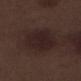Case summary:
– follow-up — total-body-photography surveillance lesion; no biopsy
– image — ~15 mm crop, total-body skin-cancer survey
– lesion size — about 5 mm
– subject — male, aged 68–72
– location — the left lower leg
– automated metrics — a mean CIELAB color near L≈21 a*≈14 b*≈15, roughly 5 lightness units darker than nearby skin, and a lesion-to-skin contrast of about 7.5 (normalized; higher = more distinct); a border-irregularity index near 1.5/10, a color-variation rating of about 2.5/10, and peripheral color asymmetry of about 0.5; a nevus-likeness score of about 25/100 and lesion-presence confidence of about 100/100